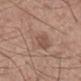Assessment:
Recorded during total-body skin imaging; not selected for excision or biopsy.
Image and clinical context:
About 8 mm across. Automated tile analysis of the lesion measured an area of roughly 13 mm² and an eccentricity of roughly 0.95. It also reported an average lesion color of about L≈54 a*≈17 b*≈26 (CIELAB), a lesion–skin lightness drop of about 7, and a normalized lesion–skin contrast near 5. The software also gave a border-irregularity rating of about 5/10 and peripheral color asymmetry of about 1.5. The software also gave a classifier nevus-likeness of about 0/100 and a lesion-detection confidence of about 70/100. From the mid back. This image is a 15 mm lesion crop taken from a total-body photograph. Captured under white-light illumination. The patient is a male approximately 60 years of age.